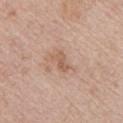<record>
  <lighting>white-light</lighting>
  <site>upper back</site>
  <automated_metrics>
    <cielab_L>58</cielab_L>
    <cielab_a>20</cielab_a>
    <cielab_b>30</cielab_b>
    <vs_skin_contrast_norm>6.0</vs_skin_contrast_norm>
    <border_irregularity_0_10>4.5</border_irregularity_0_10>
    <color_variation_0_10>3.0</color_variation_0_10>
  </automated_metrics>
  <lesion_size>
    <long_diameter_mm_approx>3.0</long_diameter_mm_approx>
  </lesion_size>
  <patient>
    <sex>female</sex>
    <age_approx>70</age_approx>
  </patient>
  <image>
    <source>total-body photography crop</source>
    <field_of_view_mm>15</field_of_view_mm>
  </image>
</record>